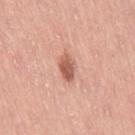Captured during whole-body skin photography for melanoma surveillance; the lesion was not biopsied. Approximately 3 mm at its widest. A close-up tile cropped from a whole-body skin photograph, about 15 mm across. A female subject aged approximately 40. The total-body-photography lesion software estimated a shape eccentricity near 0.85. It also reported radial color variation of about 1.5. Captured under white-light illumination. Located on the right thigh.